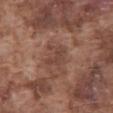Acquisition and patient details:
A male subject approximately 75 years of age. A 15 mm crop from a total-body photograph taken for skin-cancer surveillance. The lesion is on the front of the torso.